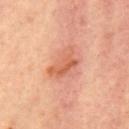notes — imaged on a skin check; not biopsied
size — about 4.5 mm
location — the mid back
tile lighting — cross-polarized illumination
patient — female, aged 43–47
imaging modality — ~15 mm tile from a whole-body skin photo